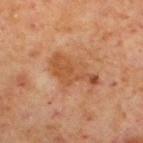Recorded during total-body skin imaging; not selected for excision or biopsy.
On the left lower leg.
A male patient about 60 years old.
Cropped from a whole-body photographic skin survey; the tile spans about 15 mm.